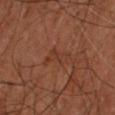Automated image analysis of the tile measured a lesion-to-skin contrast of about 4.5 (normalized; higher = more distinct). The software also gave a border-irregularity index near 5/10, a within-lesion color-variation index near 1.5/10, and a peripheral color-asymmetry measure near 0.5. And it measured a nevus-likeness score of about 0/100 and lesion-presence confidence of about 100/100.
The lesion's longest dimension is about 4 mm.
The lesion is on the right forearm.
A close-up tile cropped from a whole-body skin photograph, about 15 mm across.
A male subject approximately 70 years of age.
Imaged with cross-polarized lighting.